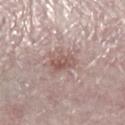Clinical impression: The lesion was photographed on a routine skin check and not biopsied; there is no pathology result. Clinical summary: The tile uses white-light illumination. Approximately 3.5 mm at its widest. A female subject roughly 70 years of age. The lesion is located on the right lower leg. A 15 mm close-up extracted from a 3D total-body photography capture.